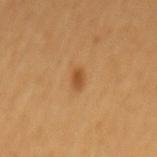workup: no biopsy performed (imaged during a skin exam) | lesion size: ≈2.5 mm | body site: the back | automated metrics: a border-irregularity index near 2/10 and peripheral color asymmetry of about 0; a classifier nevus-likeness of about 95/100 | imaging modality: 15 mm crop, total-body photography | patient: male, aged 48 to 52 | illumination: cross-polarized illumination.